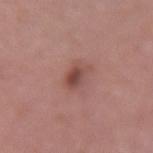Imaged during a routine full-body skin examination; the lesion was not biopsied and no histopathology is available. Automated tile analysis of the lesion measured a mean CIELAB color near L≈48 a*≈23 b*≈24, roughly 10 lightness units darker than nearby skin, and a lesion-to-skin contrast of about 7 (normalized; higher = more distinct). The analysis additionally found border irregularity of about 2.5 on a 0–10 scale, internal color variation of about 5.5 on a 0–10 scale, and a peripheral color-asymmetry measure near 1.5. This is a white-light tile. A 15 mm crop from a total-body photograph taken for skin-cancer surveillance. A female patient, roughly 50 years of age. About 3 mm across. On the right forearm.